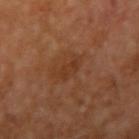Impression:
Imaged during a routine full-body skin examination; the lesion was not biopsied and no histopathology is available.
Acquisition and patient details:
The patient is a male in their mid- to late 50s. A 15 mm close-up tile from a total-body photography series done for melanoma screening. On the right upper arm. Imaged with cross-polarized lighting.